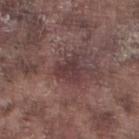anatomic site: the leg
imaging modality: ~15 mm crop, total-body skin-cancer survey
patient: male, in their mid-70s